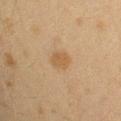From the left upper arm. The patient is a female aged 38–42. A region of skin cropped from a whole-body photographic capture, roughly 15 mm wide.